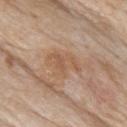Clinical impression: No biopsy was performed on this lesion — it was imaged during a full skin examination and was not determined to be concerning. Acquisition and patient details: Imaged with white-light lighting. The total-body-photography lesion software estimated an outline eccentricity of about 0.75 (0 = round, 1 = elongated) and two-axis asymmetry of about 0.45. The software also gave border irregularity of about 5.5 on a 0–10 scale, a color-variation rating of about 3/10, and a peripheral color-asymmetry measure near 1. The subject is a male aged 83 or over. Longest diameter approximately 4.5 mm. A close-up tile cropped from a whole-body skin photograph, about 15 mm across. Located on the upper back.